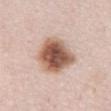- biopsy status — no biopsy performed (imaged during a skin exam)
- lighting — white-light
- automated metrics — a mean CIELAB color near L≈57 a*≈20 b*≈28, a lesion–skin lightness drop of about 18, and a lesion-to-skin contrast of about 11.5 (normalized; higher = more distinct); a classifier nevus-likeness of about 95/100 and lesion-presence confidence of about 100/100
- lesion size — about 5.5 mm
- subject — female, roughly 40 years of age
- location — the abdomen
- acquisition — ~15 mm tile from a whole-body skin photo